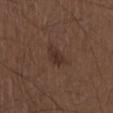Clinical impression: The lesion was tiled from a total-body skin photograph and was not biopsied. Clinical summary: The patient is a male in their 50s. This is a white-light tile. The lesion is located on the chest. A roughly 15 mm field-of-view crop from a total-body skin photograph.